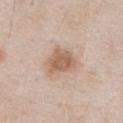site: chest
image:
  source: total-body photography crop
  field_of_view_mm: 15
lighting: white-light
patient:
  sex: male
  age_approx: 55